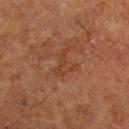Q: Where on the body is the lesion?
A: the right lower leg
Q: What is the imaging modality?
A: 15 mm crop, total-body photography
Q: Patient demographics?
A: male, approximately 65 years of age